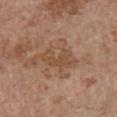Findings:
• notes · imaged on a skin check; not biopsied
• acquisition · total-body-photography crop, ~15 mm field of view
• body site · the chest
• lighting · white-light illumination
• patient · female, in their mid- to late 60s
• automated metrics · a border-irregularity index near 5.5/10 and radial color variation of about 0.5; a lesion-detection confidence of about 100/100